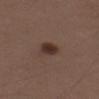- follow-up: imaged on a skin check; not biopsied
- automated metrics: a shape eccentricity near 0.75 and two-axis asymmetry of about 0.3; border irregularity of about 2.5 on a 0–10 scale
- diameter: about 3 mm
- subject: male, aged 28 to 32
- anatomic site: the right upper arm
- image source: ~15 mm tile from a whole-body skin photo